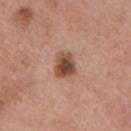Clinical impression: Imaged during a routine full-body skin examination; the lesion was not biopsied and no histopathology is available. Acquisition and patient details: A 15 mm close-up extracted from a 3D total-body photography capture. The lesion is located on the mid back. A male patient, aged approximately 70.